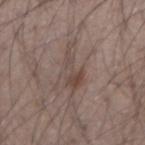biopsy status=no biopsy performed (imaged during a skin exam) | image=~15 mm crop, total-body skin-cancer survey | diameter=about 5.5 mm | subject=male, aged 43 to 47 | anatomic site=the left forearm | automated lesion analysis=an area of roughly 7 mm², an eccentricity of roughly 0.95, and two-axis asymmetry of about 0.55; a lesion color around L≈45 a*≈14 b*≈21 in CIELAB and a normalized border contrast of about 6; a border-irregularity rating of about 7.5/10 and a peripheral color-asymmetry measure near 1; an automated nevus-likeness rating near 0 out of 100 and a detector confidence of about 90 out of 100 that the crop contains a lesion.